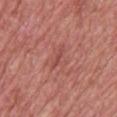workup = no biopsy performed (imaged during a skin exam)
automated metrics = about 7 CIELAB-L* units darker than the surrounding skin and a normalized lesion–skin contrast near 5; a border-irregularity rating of about 5.5/10, a within-lesion color-variation index near 0/10, and peripheral color asymmetry of about 0; a classifier nevus-likeness of about 0/100
image source = ~15 mm tile from a whole-body skin photo
subject = male, aged 58–62
lesion size = ≈2.5 mm
tile lighting = white-light
location = the back